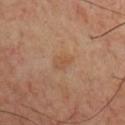Background:
A male patient aged 68–72. Automated image analysis of the tile measured a lesion area of about 3.5 mm², an eccentricity of roughly 0.75, and a symmetry-axis asymmetry near 0.3. The software also gave an average lesion color of about L≈49 a*≈19 b*≈31 (CIELAB), a lesion–skin lightness drop of about 5, and a normalized border contrast of about 5. The analysis additionally found border irregularity of about 3 on a 0–10 scale, a color-variation rating of about 1.5/10, and radial color variation of about 0.5. Cropped from a whole-body photographic skin survey; the tile spans about 15 mm. From the front of the torso. The recorded lesion diameter is about 2.5 mm. This is a cross-polarized tile.